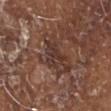Captured during whole-body skin photography for melanoma surveillance; the lesion was not biopsied. Measured at roughly 5.5 mm in maximum diameter. This is a white-light tile. A male patient, aged approximately 80. This image is a 15 mm lesion crop taken from a total-body photograph. The lesion is located on the head or neck.